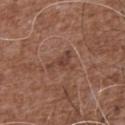Assessment:
The lesion was photographed on a routine skin check and not biopsied; there is no pathology result.
Background:
Cropped from a whole-body photographic skin survey; the tile spans about 15 mm. The lesion is on the chest. A male subject aged 73–77. Captured under white-light illumination.